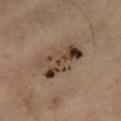Clinical impression:
Imaged during a routine full-body skin examination; the lesion was not biopsied and no histopathology is available.
Clinical summary:
Cropped from a total-body skin-imaging series; the visible field is about 15 mm. Captured under cross-polarized illumination. Located on the left leg. The lesion-visualizer software estimated an area of roughly 16 mm², an eccentricity of roughly 0.85, and two-axis asymmetry of about 0.25. The software also gave a lesion color around L≈40 a*≈13 b*≈26 in CIELAB and a normalized lesion–skin contrast near 9. The software also gave a border-irregularity index near 3.5/10, internal color variation of about 10 on a 0–10 scale, and radial color variation of about 3.5. A male subject in their mid-60s.